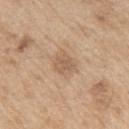The lesion was tiled from a total-body skin photograph and was not biopsied. The patient is a male aged 68–72. A 15 mm close-up tile from a total-body photography series done for melanoma screening. About 3 mm across. Located on the left upper arm.